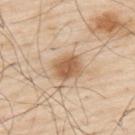workup = imaged on a skin check; not biopsied
automated lesion analysis = an area of roughly 8 mm² and a symmetry-axis asymmetry near 0.2; a lesion color around L≈60 a*≈18 b*≈35 in CIELAB and a normalized border contrast of about 8.5
lesion diameter = ≈3.5 mm
site = the upper back
subject = male, aged 78 to 82
image source = ~15 mm crop, total-body skin-cancer survey